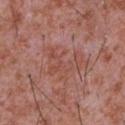biopsy_status: not biopsied; imaged during a skin examination
lighting: white-light
site: chest
lesion_size:
  long_diameter_mm_approx: 5.0
image:
  source: total-body photography crop
  field_of_view_mm: 15
patient:
  sex: male
  age_approx: 45